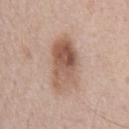Impression: Imaged during a routine full-body skin examination; the lesion was not biopsied and no histopathology is available. Acquisition and patient details: About 6 mm across. This is a white-light tile. Cropped from a total-body skin-imaging series; the visible field is about 15 mm. The total-body-photography lesion software estimated an average lesion color of about L≈56 a*≈19 b*≈28 (CIELAB), about 13 CIELAB-L* units darker than the surrounding skin, and a normalized lesion–skin contrast near 8.5. A male subject about 55 years old.